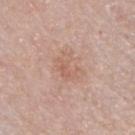notes=catalogued during a skin exam; not biopsied | subject=male, approximately 60 years of age | lighting=white-light | image source=total-body-photography crop, ~15 mm field of view | body site=the front of the torso | size=≈3.5 mm.